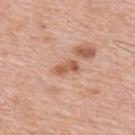The tile uses white-light illumination.
The subject is a male roughly 80 years of age.
Automated image analysis of the tile measured a footprint of about 4 mm² and a shape eccentricity near 0.85. And it measured an average lesion color of about L≈58 a*≈24 b*≈33 (CIELAB) and roughly 11 lightness units darker than nearby skin. And it measured a nevus-likeness score of about 0/100.
On the back.
Measured at roughly 3 mm in maximum diameter.
Cropped from a total-body skin-imaging series; the visible field is about 15 mm.